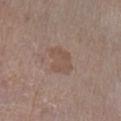Case summary:
- notes — total-body-photography surveillance lesion; no biopsy